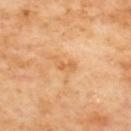{
  "image": {
    "source": "total-body photography crop",
    "field_of_view_mm": 15
  },
  "site": "upper back"
}